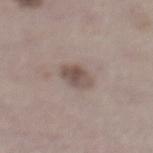image source: 15 mm crop, total-body photography; site: the left lower leg; patient: female, aged 48 to 52; size: ~3 mm (longest diameter).